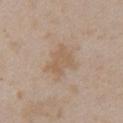{
  "biopsy_status": "not biopsied; imaged during a skin examination",
  "automated_metrics": {
    "border_irregularity_0_10": 4.0,
    "color_variation_0_10": 2.0,
    "nevus_likeness_0_100": 0,
    "lesion_detection_confidence_0_100": 100
  },
  "image": {
    "source": "total-body photography crop",
    "field_of_view_mm": 15
  },
  "lesion_size": {
    "long_diameter_mm_approx": 4.0
  },
  "site": "chest",
  "patient": {
    "sex": "female",
    "age_approx": 30
  },
  "lighting": "white-light"
}